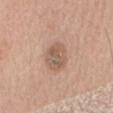Part of a total-body skin-imaging series; this lesion was reviewed on a skin check and was not flagged for biopsy. Captured under white-light illumination. A male patient, aged 58 to 62. The lesion is located on the back. Longest diameter approximately 3.5 mm. This image is a 15 mm lesion crop taken from a total-body photograph.